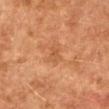The lesion was photographed on a routine skin check and not biopsied; there is no pathology result.
The lesion is on the right upper arm.
A female patient, roughly 60 years of age.
A 15 mm close-up tile from a total-body photography series done for melanoma screening.
Captured under cross-polarized illumination.